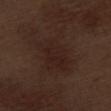follow-up: total-body-photography surveillance lesion; no biopsy
imaging modality: ~15 mm crop, total-body skin-cancer survey
patient: male, aged around 70
TBP lesion metrics: two-axis asymmetry of about 0.35; a mean CIELAB color near L≈22 a*≈15 b*≈19, about 5 CIELAB-L* units darker than the surrounding skin, and a normalized lesion–skin contrast near 6
body site: the left thigh